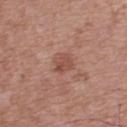workup: total-body-photography surveillance lesion; no biopsy | location: the back | lighting: white-light illumination | imaging modality: ~15 mm crop, total-body skin-cancer survey | subject: male, aged 63 to 67.